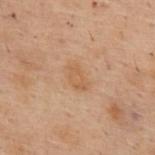follow-up: catalogued during a skin exam; not biopsied
automated lesion analysis: a footprint of about 6 mm², an eccentricity of roughly 0.8, and a symmetry-axis asymmetry near 0.25; a mean CIELAB color near L≈48 a*≈16 b*≈30, roughly 5 lightness units darker than nearby skin, and a lesion-to-skin contrast of about 4.5 (normalized; higher = more distinct); a nevus-likeness score of about 0/100 and a lesion-detection confidence of about 100/100
imaging modality: ~15 mm tile from a whole-body skin photo
lesion diameter: about 3.5 mm
location: the upper back
patient: female, aged 53–57
illumination: cross-polarized